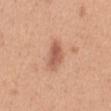Case summary:
* biopsy status: catalogued during a skin exam; not biopsied
* body site: the abdomen
* subject: female, aged 28–32
* image source: ~15 mm tile from a whole-body skin photo
* tile lighting: white-light illumination
* automated lesion analysis: a lesion color around L≈58 a*≈25 b*≈31 in CIELAB, about 12 CIELAB-L* units darker than the surrounding skin, and a normalized border contrast of about 7.5; an automated nevus-likeness rating near 85 out of 100 and a lesion-detection confidence of about 100/100
* diameter: ~4 mm (longest diameter)